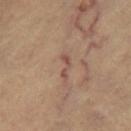This is a cross-polarized tile.
From the right thigh.
A female subject, aged 63–67.
This image is a 15 mm lesion crop taken from a total-body photograph.
The total-body-photography lesion software estimated a lesion area of about 2.5 mm² and two-axis asymmetry of about 0.55. And it measured a lesion–skin lightness drop of about 8 and a normalized border contrast of about 7. The software also gave an automated nevus-likeness rating near 0 out of 100 and a lesion-detection confidence of about 55/100.